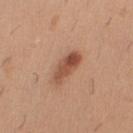The lesion was tiled from a total-body skin photograph and was not biopsied. Located on the right upper arm. A male patient, in their 40s. Measured at roughly 4 mm in maximum diameter. This is a white-light tile. A 15 mm close-up tile from a total-body photography series done for melanoma screening. The total-body-photography lesion software estimated an average lesion color of about L≈51 a*≈24 b*≈31 (CIELAB), about 12 CIELAB-L* units darker than the surrounding skin, and a lesion-to-skin contrast of about 8.5 (normalized; higher = more distinct). The software also gave an automated nevus-likeness rating near 95 out of 100.